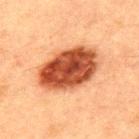Q: Was a biopsy performed?
A: catalogued during a skin exam; not biopsied
Q: How was the tile lit?
A: cross-polarized illumination
Q: How large is the lesion?
A: ~7.5 mm (longest diameter)
Q: Where on the body is the lesion?
A: the upper back
Q: What is the imaging modality?
A: total-body-photography crop, ~15 mm field of view
Q: Patient demographics?
A: male, about 50 years old